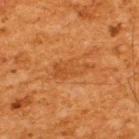Impression:
Recorded during total-body skin imaging; not selected for excision or biopsy.
Context:
A 15 mm crop from a total-body photograph taken for skin-cancer surveillance. Imaged with cross-polarized lighting. The lesion is located on the back. Longest diameter approximately 4.5 mm. A male patient, aged 63 to 67.